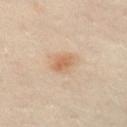The lesion was tiled from a total-body skin photograph and was not biopsied. The lesion is on the right leg. A female subject, aged 38–42. Cropped from a whole-body photographic skin survey; the tile spans about 15 mm.